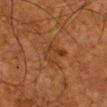Clinical impression: Recorded during total-body skin imaging; not selected for excision or biopsy. Background: Cropped from a whole-body photographic skin survey; the tile spans about 15 mm. Located on the right lower leg. Measured at roughly 3.5 mm in maximum diameter. A male patient, about 80 years old. This is a cross-polarized tile.